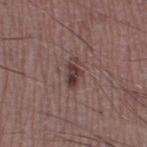biopsy_status: not biopsied; imaged during a skin examination
site: right thigh
automated_metrics:
  area_mm2_approx: 4.5
  eccentricity: 0.85
  shape_asymmetry: 0.3
  border_irregularity_0_10: 3.5
  color_variation_0_10: 3.5
  peripheral_color_asymmetry: 1.0
  lesion_detection_confidence_0_100: 100
lesion_size:
  long_diameter_mm_approx: 3.5
patient:
  sex: male
  age_approx: 50
image:
  source: total-body photography crop
  field_of_view_mm: 15